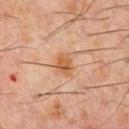<lesion>
<biopsy_status>not biopsied; imaged during a skin examination</biopsy_status>
<lesion_size>
  <long_diameter_mm_approx>3.0</long_diameter_mm_approx>
</lesion_size>
<patient>
  <sex>male</sex>
  <age_approx>60</age_approx>
</patient>
<site>chest</site>
<image>
  <source>total-body photography crop</source>
  <field_of_view_mm>15</field_of_view_mm>
</image>
</lesion>